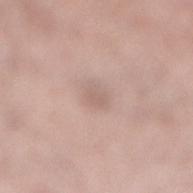Clinical summary: This is a white-light tile. Measured at roughly 3 mm in maximum diameter. A female patient, approximately 50 years of age. A region of skin cropped from a whole-body photographic capture, roughly 15 mm wide. Located on the leg.